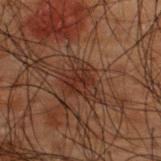Impression: The lesion was photographed on a routine skin check and not biopsied; there is no pathology result. Context: The lesion's longest dimension is about 4.5 mm. A lesion tile, about 15 mm wide, cut from a 3D total-body photograph. The lesion is on the upper back. This is a cross-polarized tile. The patient is a male in their 50s.